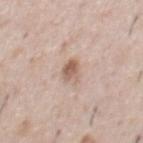Clinical summary: The lesion is located on the front of the torso. A lesion tile, about 15 mm wide, cut from a 3D total-body photograph. A male subject roughly 40 years of age.